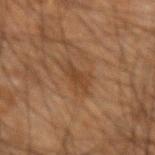This lesion was catalogued during total-body skin photography and was not selected for biopsy.
The lesion-visualizer software estimated an area of roughly 5 mm² and two-axis asymmetry of about 0.3. The analysis additionally found a border-irregularity index near 4/10, a within-lesion color-variation index near 2/10, and radial color variation of about 0.5. It also reported an automated nevus-likeness rating near 0 out of 100 and a detector confidence of about 100 out of 100 that the crop contains a lesion.
From the left forearm.
Captured under cross-polarized illumination.
A male patient, aged around 65.
The lesion's longest dimension is about 3 mm.
A 15 mm close-up tile from a total-body photography series done for melanoma screening.